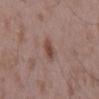Impression: The lesion was photographed on a routine skin check and not biopsied; there is no pathology result. Image and clinical context: A 15 mm close-up tile from a total-body photography series done for melanoma screening. The patient is a male aged 48–52. Imaged with white-light lighting. The lesion is on the mid back. The lesion-visualizer software estimated a lesion area of about 3.5 mm², a shape eccentricity near 0.8, and a symmetry-axis asymmetry near 0.2. It also reported a mean CIELAB color near L≈46 a*≈20 b*≈24, a lesion–skin lightness drop of about 10, and a normalized lesion–skin contrast near 8. It also reported a border-irregularity index near 2/10, a color-variation rating of about 3/10, and a peripheral color-asymmetry measure near 1. The software also gave a classifier nevus-likeness of about 70/100 and a lesion-detection confidence of about 100/100.